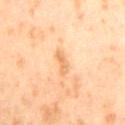The lesion was photographed on a routine skin check and not biopsied; there is no pathology result.
This is a cross-polarized tile.
A male subject aged approximately 65.
About 3 mm across.
Automated image analysis of the tile measured a lesion area of about 3 mm². And it measured an average lesion color of about L≈72 a*≈23 b*≈43 (CIELAB), a lesion–skin lightness drop of about 9, and a normalized border contrast of about 6.5.
A lesion tile, about 15 mm wide, cut from a 3D total-body photograph.